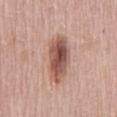Notes:
– biopsy status: imaged on a skin check; not biopsied
– automated metrics: a mean CIELAB color near L≈53 a*≈21 b*≈26 and a normalized lesion–skin contrast near 10; a border-irregularity index near 2.5/10, a color-variation rating of about 7.5/10, and a peripheral color-asymmetry measure near 2.5; a nevus-likeness score of about 95/100 and a detector confidence of about 100 out of 100 that the crop contains a lesion
– patient: female, aged approximately 60
– site: the mid back
– image: total-body-photography crop, ~15 mm field of view